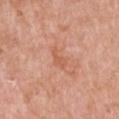This lesion was catalogued during total-body skin photography and was not selected for biopsy. An algorithmic analysis of the crop reported a border-irregularity index near 4/10 and a peripheral color-asymmetry measure near 0. A female patient, aged around 70. Located on the front of the torso. A 15 mm close-up tile from a total-body photography series done for melanoma screening. The tile uses white-light illumination. The lesion's longest dimension is about 2.5 mm.